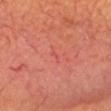{
  "biopsy_status": "not biopsied; imaged during a skin examination",
  "site": "head or neck",
  "image": {
    "source": "total-body photography crop",
    "field_of_view_mm": 15
  },
  "patient": {
    "sex": "male",
    "age_approx": 70
  },
  "lesion_size": {
    "long_diameter_mm_approx": 1.5
  },
  "automated_metrics": {
    "area_mm2_approx": 1.0,
    "eccentricity": 0.9,
    "shape_asymmetry": 0.5,
    "cielab_L": 53,
    "cielab_a": 36,
    "cielab_b": 30,
    "vs_skin_darker_L": 5.0,
    "vs_skin_contrast_norm": 3.0
  }
}